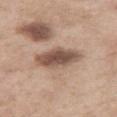This lesion was catalogued during total-body skin photography and was not selected for biopsy. An algorithmic analysis of the crop reported a lesion color around L≈52 a*≈17 b*≈25 in CIELAB, about 15 CIELAB-L* units darker than the surrounding skin, and a lesion-to-skin contrast of about 10 (normalized; higher = more distinct). It also reported a border-irregularity index near 2/10 and radial color variation of about 1.5. It also reported a lesion-detection confidence of about 100/100. A roughly 15 mm field-of-view crop from a total-body skin photograph. A female subject approximately 55 years of age. The lesion is located on the right thigh.